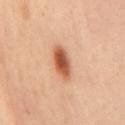{"biopsy_status": "not biopsied; imaged during a skin examination", "site": "back", "patient": {"sex": "male", "age_approx": 45}, "image": {"source": "total-body photography crop", "field_of_view_mm": 15}, "lighting": "cross-polarized", "automated_metrics": {"cielab_L": 56, "cielab_a": 26, "cielab_b": 36, "vs_skin_contrast_norm": 10.5, "nevus_likeness_0_100": 100, "lesion_detection_confidence_0_100": 100}}